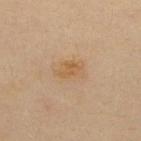notes: imaged on a skin check; not biopsied | patient: male, aged approximately 35 | size: about 3.5 mm | anatomic site: the upper back | image source: ~15 mm tile from a whole-body skin photo | tile lighting: cross-polarized illumination.